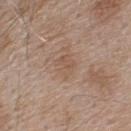The lesion was photographed on a routine skin check and not biopsied; there is no pathology result.
Longest diameter approximately 2.5 mm.
On the back.
Captured under white-light illumination.
An algorithmic analysis of the crop reported a lesion area of about 4.5 mm², an outline eccentricity of about 0.5 (0 = round, 1 = elongated), and two-axis asymmetry of about 0.25. The software also gave a mean CIELAB color near L≈54 a*≈17 b*≈28, about 6 CIELAB-L* units darker than the surrounding skin, and a normalized border contrast of about 4.5. It also reported a classifier nevus-likeness of about 0/100 and a lesion-detection confidence of about 100/100.
A male subject roughly 55 years of age.
A region of skin cropped from a whole-body photographic capture, roughly 15 mm wide.